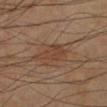  biopsy_status: not biopsied; imaged during a skin examination
  automated_metrics:
    border_irregularity_0_10: 4.0
    nevus_likeness_0_100: 35
    lesion_detection_confidence_0_100: 100
  image:
    source: total-body photography crop
    field_of_view_mm: 15
  lesion_size:
    long_diameter_mm_approx: 3.5
  site: left lower leg
  patient:
    sex: male
    age_approx: 70
  lighting: cross-polarized